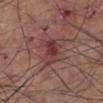follow-up — total-body-photography surveillance lesion; no biopsy
patient — male, in their mid-40s
image — ~15 mm crop, total-body skin-cancer survey
body site — the left lower leg
diameter — ≈4 mm
tile lighting — white-light